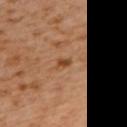  biopsy_status: not biopsied; imaged during a skin examination
  site: mid back
  patient:
    sex: female
    age_approx: 55
  lesion_size:
    long_diameter_mm_approx: 2.0
  image:
    source: total-body photography crop
    field_of_view_mm: 15
  automated_metrics:
    area_mm2_approx: 2.5
    eccentricity: 0.75
    shape_asymmetry: 0.4
    border_irregularity_0_10: 3.5
    color_variation_0_10: 3.5